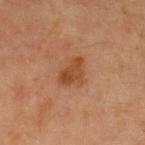Assessment: Part of a total-body skin-imaging series; this lesion was reviewed on a skin check and was not flagged for biopsy. Context: On the left upper arm. Approximately 3.5 mm at its widest. A female subject in their 70s. Automated image analysis of the tile measured a border-irregularity index near 3.5/10, a within-lesion color-variation index near 3.5/10, and radial color variation of about 1. And it measured an automated nevus-likeness rating near 85 out of 100 and a lesion-detection confidence of about 100/100. A region of skin cropped from a whole-body photographic capture, roughly 15 mm wide. The tile uses cross-polarized illumination.